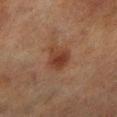* biopsy status: total-body-photography surveillance lesion; no biopsy
* automated lesion analysis: a lesion area of about 8 mm², an eccentricity of roughly 0.65, and two-axis asymmetry of about 0.3; a border-irregularity rating of about 3/10 and a peripheral color-asymmetry measure near 1; a lesion-detection confidence of about 100/100
* lesion size: about 3.5 mm
* location: the leg
* tile lighting: cross-polarized illumination
* image source: ~15 mm crop, total-body skin-cancer survey
* patient: male, aged around 70